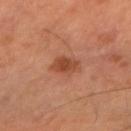The lesion was photographed on a routine skin check and not biopsied; there is no pathology result. The lesion-visualizer software estimated a classifier nevus-likeness of about 90/100 and a lesion-detection confidence of about 100/100. This image is a 15 mm lesion crop taken from a total-body photograph. Measured at roughly 3.5 mm in maximum diameter. A male subject roughly 60 years of age. Imaged with cross-polarized lighting. From the right forearm.